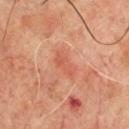- biopsy status · catalogued during a skin exam; not biopsied
- acquisition · ~15 mm crop, total-body skin-cancer survey
- patient · male, aged 68 to 72
- site · the chest